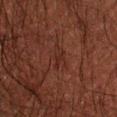This lesion was catalogued during total-body skin photography and was not selected for biopsy.
A region of skin cropped from a whole-body photographic capture, roughly 15 mm wide.
A male subject, about 50 years old.
From the right forearm.
This is a cross-polarized tile.